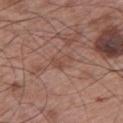follow-up = no biopsy performed (imaged during a skin exam)
automated lesion analysis = an average lesion color of about L≈47 a*≈21 b*≈26 (CIELAB) and a normalized lesion–skin contrast near 5.5; border irregularity of about 5.5 on a 0–10 scale, a within-lesion color-variation index near 1/10, and peripheral color asymmetry of about 0.5; a classifier nevus-likeness of about 0/100 and lesion-presence confidence of about 90/100
lesion diameter = about 3 mm
imaging modality = ~15 mm crop, total-body skin-cancer survey
subject = male, aged 63–67
lighting = white-light illumination
body site = the left upper arm